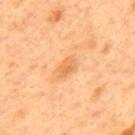Clinical impression: Imaged during a routine full-body skin examination; the lesion was not biopsied and no histopathology is available. Context: The total-body-photography lesion software estimated an area of roughly 3 mm², an eccentricity of roughly 0.8, and a shape-asymmetry score of about 0.35 (0 = symmetric). And it measured an average lesion color of about L≈68 a*≈26 b*≈47 (CIELAB), roughly 9 lightness units darker than nearby skin, and a lesion-to-skin contrast of about 6 (normalized; higher = more distinct). It also reported border irregularity of about 3 on a 0–10 scale, internal color variation of about 3 on a 0–10 scale, and radial color variation of about 1. Cropped from a total-body skin-imaging series; the visible field is about 15 mm. The lesion is located on the mid back. The subject is a male aged 48–52.